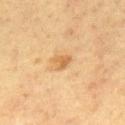workup: catalogued during a skin exam; not biopsied
imaging modality: ~15 mm crop, total-body skin-cancer survey
illumination: cross-polarized
anatomic site: the upper back
automated metrics: border irregularity of about 3.5 on a 0–10 scale, a color-variation rating of about 2.5/10, and radial color variation of about 1; a classifier nevus-likeness of about 45/100 and a detector confidence of about 100 out of 100 that the crop contains a lesion
subject: male, in their mid- to late 60s
lesion diameter: ~2.5 mm (longest diameter)